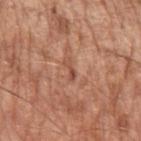Findings:
* workup — no biopsy performed (imaged during a skin exam)
* imaging modality — total-body-photography crop, ~15 mm field of view
* anatomic site — the left upper arm
* subject — male, in their mid- to late 60s
* image-analysis metrics — a lesion area of about 2 mm², an outline eccentricity of about 0.95 (0 = round, 1 = elongated), and a shape-asymmetry score of about 0.55 (0 = symmetric)
* size — ≈2.5 mm
* illumination — white-light illumination